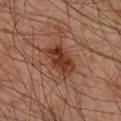Q: Was a biopsy performed?
A: catalogued during a skin exam; not biopsied
Q: Lesion location?
A: the right forearm
Q: What is the imaging modality?
A: ~15 mm crop, total-body skin-cancer survey
Q: How large is the lesion?
A: about 4.5 mm
Q: What are the patient's age and sex?
A: male, aged 43–47